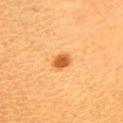{"lesion_size": {"long_diameter_mm_approx": 2.5}, "lighting": "cross-polarized", "site": "chest", "patient": {"sex": "female", "age_approx": 30}, "image": {"source": "total-body photography crop", "field_of_view_mm": 15}}